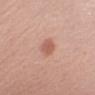automated lesion analysis — internal color variation of about 1.5 on a 0–10 scale and radial color variation of about 0.5; a classifier nevus-likeness of about 90/100 and a lesion-detection confidence of about 100/100 | anatomic site — the right upper arm | subject — female, aged around 50 | image source — total-body-photography crop, ~15 mm field of view.